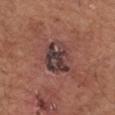Clinical impression: No biopsy was performed on this lesion — it was imaged during a full skin examination and was not determined to be concerning. Clinical summary: Captured under white-light illumination. A lesion tile, about 15 mm wide, cut from a 3D total-body photograph. On the left forearm. A male subject, aged approximately 70. The lesion-visualizer software estimated a lesion area of about 11 mm² and two-axis asymmetry of about 0.2. The software also gave a lesion color around L≈38 a*≈18 b*≈18 in CIELAB. The analysis additionally found a border-irregularity rating of about 2/10. The recorded lesion diameter is about 4 mm.